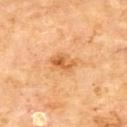Q: Is there a histopathology result?
A: catalogued during a skin exam; not biopsied
Q: What lighting was used for the tile?
A: cross-polarized illumination
Q: What are the patient's age and sex?
A: male, aged 68–72
Q: What is the anatomic site?
A: the back
Q: How large is the lesion?
A: ≈3.5 mm
Q: How was this image acquired?
A: ~15 mm tile from a whole-body skin photo
Q: Automated lesion metrics?
A: a border-irregularity rating of about 3.5/10, internal color variation of about 5 on a 0–10 scale, and radial color variation of about 2; an automated nevus-likeness rating near 15 out of 100 and lesion-presence confidence of about 100/100